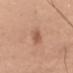This lesion was catalogued during total-body skin photography and was not selected for biopsy. The lesion-visualizer software estimated a footprint of about 4 mm², a shape eccentricity near 0.85, and a symmetry-axis asymmetry near 0.2. It also reported a mean CIELAB color near L≈56 a*≈23 b*≈32, about 10 CIELAB-L* units darker than the surrounding skin, and a lesion-to-skin contrast of about 6.5 (normalized; higher = more distinct). The lesion's longest dimension is about 3 mm. The lesion is on the left upper arm. A 15 mm close-up tile from a total-body photography series done for melanoma screening. The subject is a male approximately 75 years of age.